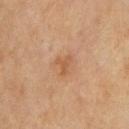Imaged during a routine full-body skin examination; the lesion was not biopsied and no histopathology is available. A male patient aged 63 to 67. Captured under cross-polarized illumination. The total-body-photography lesion software estimated a lesion area of about 3 mm², a shape eccentricity near 0.6, and a shape-asymmetry score of about 0.65 (0 = symmetric). It also reported an average lesion color of about L≈53 a*≈22 b*≈35 (CIELAB), a lesion–skin lightness drop of about 7, and a lesion-to-skin contrast of about 6 (normalized; higher = more distinct). It also reported a classifier nevus-likeness of about 30/100. The lesion is on the chest. A roughly 15 mm field-of-view crop from a total-body skin photograph.